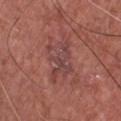On the chest.
Imaged with white-light lighting.
Cropped from a whole-body photographic skin survey; the tile spans about 15 mm.
The recorded lesion diameter is about 5 mm.
A male subject, approximately 75 years of age.
The total-body-photography lesion software estimated an area of roughly 11 mm², an outline eccentricity of about 0.8 (0 = round, 1 = elongated), and a shape-asymmetry score of about 0.35 (0 = symmetric). And it measured an average lesion color of about L≈44 a*≈24 b*≈21 (CIELAB) and a normalized lesion–skin contrast near 6. And it measured border irregularity of about 6.5 on a 0–10 scale, internal color variation of about 3.5 on a 0–10 scale, and a peripheral color-asymmetry measure near 1.